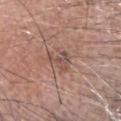notes: catalogued during a skin exam; not biopsied | illumination: white-light | lesion size: ~3 mm (longest diameter) | acquisition: total-body-photography crop, ~15 mm field of view | patient: male, aged around 80 | body site: the head or neck | image-analysis metrics: a footprint of about 5.5 mm², an eccentricity of roughly 0.4, and two-axis asymmetry of about 0.55; a classifier nevus-likeness of about 0/100.